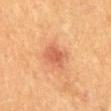Case summary:
• workup · no biopsy performed (imaged during a skin exam)